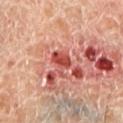Q: Was this lesion biopsied?
A: total-body-photography surveillance lesion; no biopsy
Q: Automated lesion metrics?
A: a classifier nevus-likeness of about 0/100 and a detector confidence of about 60 out of 100 that the crop contains a lesion
Q: What is the lesion's diameter?
A: about 3 mm
Q: How was this image acquired?
A: 15 mm crop, total-body photography
Q: Patient demographics?
A: male, about 55 years old
Q: Illumination type?
A: cross-polarized illumination
Q: Lesion location?
A: the left lower leg